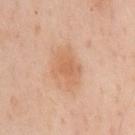Part of a total-body skin-imaging series; this lesion was reviewed on a skin check and was not flagged for biopsy.
Captured under cross-polarized illumination.
This image is a 15 mm lesion crop taken from a total-body photograph.
A male subject, aged 53 to 57.
On the mid back.
Automated tile analysis of the lesion measured roughly 8 lightness units darker than nearby skin and a normalized border contrast of about 6. The software also gave a border-irregularity rating of about 3.5/10 and a within-lesion color-variation index near 2.5/10.